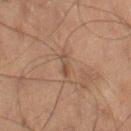Case summary:
• tile lighting · cross-polarized
• image source · ~15 mm crop, total-body skin-cancer survey
• patient · male, about 70 years old
• size · ≈3 mm
• anatomic site · the right thigh
• automated lesion analysis · an area of roughly 2.5 mm² and a symmetry-axis asymmetry near 0.4; a lesion color around L≈37 a*≈14 b*≈22 in CIELAB, roughly 6 lightness units darker than nearby skin, and a normalized border contrast of about 5.5; a color-variation rating of about 0/10 and peripheral color asymmetry of about 0; an automated nevus-likeness rating near 0 out of 100